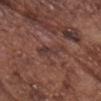Assessment: Part of a total-body skin-imaging series; this lesion was reviewed on a skin check and was not flagged for biopsy. Context: Automated tile analysis of the lesion measured an area of roughly 5.5 mm² and a symmetry-axis asymmetry near 0.4. The software also gave border irregularity of about 4 on a 0–10 scale, a color-variation rating of about 3.5/10, and peripheral color asymmetry of about 1. Imaged with white-light lighting. The patient is a male aged around 75. The lesion is located on the head or neck. A roughly 15 mm field-of-view crop from a total-body skin photograph.